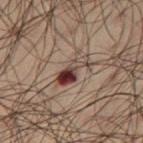The lesion was photographed on a routine skin check and not biopsied; there is no pathology result.
A male subject aged around 50.
A roughly 15 mm field-of-view crop from a total-body skin photograph.
The tile uses cross-polarized illumination.
The lesion is located on the right thigh.